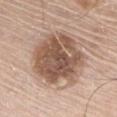Q: How large is the lesion?
A: ~7 mm (longest diameter)
Q: How was this image acquired?
A: ~15 mm crop, total-body skin-cancer survey
Q: Illumination type?
A: white-light
Q: Lesion location?
A: the chest
Q: Automated lesion metrics?
A: a lesion area of about 34 mm² and two-axis asymmetry of about 0.15; roughly 15 lightness units darker than nearby skin and a normalized lesion–skin contrast near 9.5; a border-irregularity rating of about 2.5/10, a within-lesion color-variation index near 6/10, and radial color variation of about 2
Q: Who is the patient?
A: male, in their mid-60s